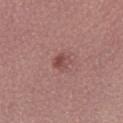{"biopsy_status": "not biopsied; imaged during a skin examination", "image": {"source": "total-body photography crop", "field_of_view_mm": 15}, "patient": {"sex": "female", "age_approx": 20}, "site": "right lower leg", "lighting": "white-light", "automated_metrics": {"area_mm2_approx": 3.5, "eccentricity": 0.35, "shape_asymmetry": 0.2, "cielab_L": 47, "cielab_a": 24, "cielab_b": 22, "vs_skin_darker_L": 9.0, "vs_skin_contrast_norm": 6.5, "border_irregularity_0_10": 2.0, "color_variation_0_10": 3.5, "peripheral_color_asymmetry": 1.0}, "lesion_size": {"long_diameter_mm_approx": 2.0}}